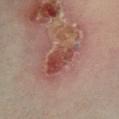workup = imaged on a skin check; not biopsied
patient = female, aged 33 to 37
anatomic site = the right lower leg
image source = 15 mm crop, total-body photography